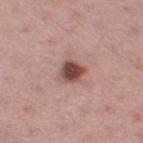Captured during whole-body skin photography for melanoma surveillance; the lesion was not biopsied. About 3 mm across. The lesion is located on the right thigh. A 15 mm close-up extracted from a 3D total-body photography capture. This is a white-light tile. A female patient aged approximately 35.